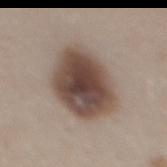Part of a total-body skin-imaging series; this lesion was reviewed on a skin check and was not flagged for biopsy.
The patient is a male aged approximately 30.
The tile uses white-light illumination.
A roughly 15 mm field-of-view crop from a total-body skin photograph.
The total-body-photography lesion software estimated a lesion area of about 30 mm² and a shape eccentricity near 0.6. The analysis additionally found a border-irregularity index near 1.5/10, internal color variation of about 6.5 on a 0–10 scale, and a peripheral color-asymmetry measure near 1.5.
Located on the mid back.
Approximately 7 mm at its widest.